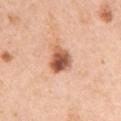This lesion was catalogued during total-body skin photography and was not selected for biopsy.
The lesion-visualizer software estimated a footprint of about 8 mm², an eccentricity of roughly 0.4, and a shape-asymmetry score of about 0.3 (0 = symmetric). The analysis additionally found border irregularity of about 3 on a 0–10 scale, a color-variation rating of about 9/10, and peripheral color asymmetry of about 3. The analysis additionally found a classifier nevus-likeness of about 95/100 and lesion-presence confidence of about 100/100.
On the left upper arm.
The subject is a female aged 58 to 62.
This is a white-light tile.
A region of skin cropped from a whole-body photographic capture, roughly 15 mm wide.